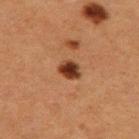Impression: Captured during whole-body skin photography for melanoma surveillance; the lesion was not biopsied. Context: The tile uses cross-polarized illumination. The lesion's longest dimension is about 2.5 mm. The subject is a female about 50 years old. A lesion tile, about 15 mm wide, cut from a 3D total-body photograph. The total-body-photography lesion software estimated an area of roughly 4.5 mm², an outline eccentricity of about 0.6 (0 = round, 1 = elongated), and two-axis asymmetry of about 0.15. The software also gave a classifier nevus-likeness of about 100/100. The lesion is located on the left thigh.